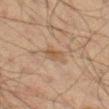Case summary:
• notes — catalogued during a skin exam; not biopsied
• tile lighting — cross-polarized
• image source — total-body-photography crop, ~15 mm field of view
• subject — male, aged 58–62
• TBP lesion metrics — roughly 6 lightness units darker than nearby skin and a normalized border contrast of about 6; lesion-presence confidence of about 100/100
• body site — the left thigh
• diameter — ~2.5 mm (longest diameter)